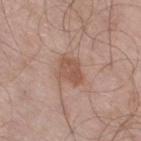Imaged during a routine full-body skin examination; the lesion was not biopsied and no histopathology is available. Longest diameter approximately 3.5 mm. Captured under white-light illumination. The subject is a male roughly 70 years of age. Cropped from a total-body skin-imaging series; the visible field is about 15 mm. The lesion-visualizer software estimated a footprint of about 8 mm² and a shape-asymmetry score of about 0.25 (0 = symmetric). The analysis additionally found a border-irregularity rating of about 2.5/10, a color-variation rating of about 2.5/10, and a peripheral color-asymmetry measure near 1. It also reported a nevus-likeness score of about 20/100 and lesion-presence confidence of about 100/100. From the right thigh.